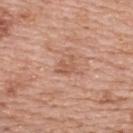The lesion was photographed on a routine skin check and not biopsied; there is no pathology result.
The lesion-visualizer software estimated a classifier nevus-likeness of about 0/100 and a lesion-detection confidence of about 100/100.
A 15 mm close-up tile from a total-body photography series done for melanoma screening.
The tile uses white-light illumination.
The subject is a female in their 60s.
The lesion is located on the upper back.
The recorded lesion diameter is about 2.5 mm.